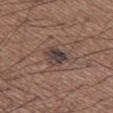notes: imaged on a skin check; not biopsied | tile lighting: white-light | patient: male, about 65 years old | imaging modality: 15 mm crop, total-body photography | size: ~3 mm (longest diameter) | body site: the left thigh.